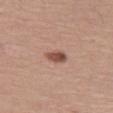biopsy status: total-body-photography surveillance lesion; no biopsy | body site: the left thigh | subject: female, aged 48 to 52 | tile lighting: white-light illumination | TBP lesion metrics: an area of roughly 4 mm², an eccentricity of roughly 0.75, and a shape-asymmetry score of about 0.25 (0 = symmetric); a border-irregularity index near 2/10, internal color variation of about 4.5 on a 0–10 scale, and peripheral color asymmetry of about 1.5; an automated nevus-likeness rating near 95 out of 100 | imaging modality: ~15 mm tile from a whole-body skin photo | size: ~2.5 mm (longest diameter).